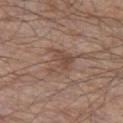| key | value |
|---|---|
| workup | no biopsy performed (imaged during a skin exam) |
| illumination | white-light illumination |
| location | the left thigh |
| image-analysis metrics | a lesion area of about 7 mm², an outline eccentricity of about 0.35 (0 = round, 1 = elongated), and a shape-asymmetry score of about 0.55 (0 = symmetric); an average lesion color of about L≈47 a*≈17 b*≈25 (CIELAB) and a lesion-to-skin contrast of about 6 (normalized; higher = more distinct); a border-irregularity rating of about 6.5/10, a color-variation rating of about 3.5/10, and radial color variation of about 1; a lesion-detection confidence of about 100/100 |
| acquisition | ~15 mm crop, total-body skin-cancer survey |
| lesion diameter | ~3 mm (longest diameter) |
| subject | male, aged around 80 |